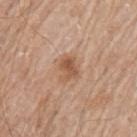Automated image analysis of the tile measured a lesion area of about 5 mm² and a shape-asymmetry score of about 0.35 (0 = symmetric). The analysis additionally found a mean CIELAB color near L≈55 a*≈21 b*≈32. The analysis additionally found an automated nevus-likeness rating near 65 out of 100. On the left upper arm. A male subject approximately 65 years of age. Cropped from a total-body skin-imaging series; the visible field is about 15 mm. The recorded lesion diameter is about 3 mm.